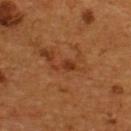<tbp_lesion>
<biopsy_status>not biopsied; imaged during a skin examination</biopsy_status>
<image>
  <source>total-body photography crop</source>
  <field_of_view_mm>15</field_of_view_mm>
</image>
<patient>
  <sex>male</sex>
  <age_approx>55</age_approx>
</patient>
<site>upper back</site>
<lighting>cross-polarized</lighting>
<automated_metrics>
  <area_mm2_approx>3.0</area_mm2_approx>
  <eccentricity>0.9</eccentricity>
  <shape_asymmetry>0.5</shape_asymmetry>
  <border_irregularity_0_10>5.0</border_irregularity_0_10>
  <color_variation_0_10>1.5</color_variation_0_10>
  <nevus_likeness_0_100>0</nevus_likeness_0_100>
  <lesion_detection_confidence_0_100>100</lesion_detection_confidence_0_100>
</automated_metrics>
<lesion_size>
  <long_diameter_mm_approx>3.0</long_diameter_mm_approx>
</lesion_size>
</tbp_lesion>